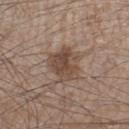This lesion was catalogued during total-body skin photography and was not selected for biopsy.
Located on the right lower leg.
Cropped from a whole-body photographic skin survey; the tile spans about 15 mm.
The patient is a male aged 43–47.